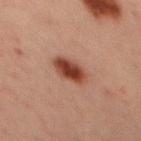biopsy status: imaged on a skin check; not biopsied
anatomic site: the mid back
tile lighting: cross-polarized
acquisition: 15 mm crop, total-body photography
patient: male, aged around 30